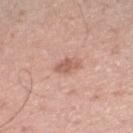Case summary:
* follow-up · catalogued during a skin exam; not biopsied
* illumination · white-light illumination
* site · the right lower leg
* patient · male, aged 48–52
* image source · 15 mm crop, total-body photography
* size · ≈2.5 mm
* automated metrics · a lesion color around L≈59 a*≈23 b*≈27 in CIELAB, a lesion–skin lightness drop of about 10, and a lesion-to-skin contrast of about 7 (normalized; higher = more distinct); a nevus-likeness score of about 10/100 and lesion-presence confidence of about 100/100